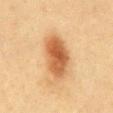Imaged with cross-polarized lighting. A 15 mm crop from a total-body photograph taken for skin-cancer surveillance. On the mid back. A female subject, in their mid-50s.